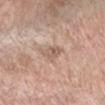The lesion was tiled from a total-body skin photograph and was not biopsied. The patient is a female roughly 55 years of age. A region of skin cropped from a whole-body photographic capture, roughly 15 mm wide. On the arm. About 3 mm across.